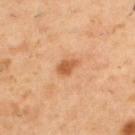The lesion was photographed on a routine skin check and not biopsied; there is no pathology result. The lesion's longest dimension is about 2.5 mm. The patient is a male aged 53–57. Imaged with cross-polarized lighting. Automated tile analysis of the lesion measured a lesion color around L≈56 a*≈25 b*≈39 in CIELAB, a lesion–skin lightness drop of about 11, and a lesion-to-skin contrast of about 7.5 (normalized; higher = more distinct). It also reported a border-irregularity index near 2.5/10 and radial color variation of about 0.5. Located on the upper back. This image is a 15 mm lesion crop taken from a total-body photograph.